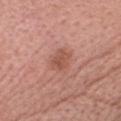Q: Was this lesion biopsied?
A: catalogued during a skin exam; not biopsied
Q: Patient demographics?
A: female, aged around 65
Q: What did automated image analysis measure?
A: an area of roughly 5.5 mm² and a shape eccentricity near 0.7; a mean CIELAB color near L≈53 a*≈25 b*≈29, about 9 CIELAB-L* units darker than the surrounding skin, and a lesion-to-skin contrast of about 6.5 (normalized; higher = more distinct); a border-irregularity index near 2/10, internal color variation of about 2.5 on a 0–10 scale, and radial color variation of about 1; a nevus-likeness score of about 25/100
Q: What is the anatomic site?
A: the head or neck
Q: How was this image acquired?
A: 15 mm crop, total-body photography